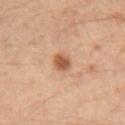Clinical impression: The lesion was tiled from a total-body skin photograph and was not biopsied. Background: The lesion is on the arm. A lesion tile, about 15 mm wide, cut from a 3D total-body photograph. A female patient aged 53–57. The lesion's longest dimension is about 2.5 mm.